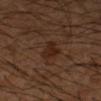workup: imaged on a skin check; not biopsied | subject: male, aged approximately 55 | image source: ~15 mm tile from a whole-body skin photo | site: the right forearm.